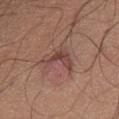No biopsy was performed on this lesion — it was imaged during a full skin examination and was not determined to be concerning. The lesion's longest dimension is about 4 mm. A male patient, aged 63 to 67. A close-up tile cropped from a whole-body skin photograph, about 15 mm across. Automated tile analysis of the lesion measured a footprint of about 6.5 mm² and a shape-asymmetry score of about 0.65 (0 = symmetric). The analysis additionally found a border-irregularity rating of about 7/10 and a within-lesion color-variation index near 3.5/10. It also reported an automated nevus-likeness rating near 35 out of 100 and a lesion-detection confidence of about 60/100. Imaged with white-light lighting. Located on the abdomen.